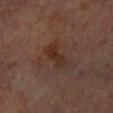This lesion was catalogued during total-body skin photography and was not selected for biopsy. Approximately 4 mm at its widest. A 15 mm close-up extracted from a 3D total-body photography capture. A male subject approximately 65 years of age. The tile uses cross-polarized illumination. The lesion is located on the left forearm. Automated tile analysis of the lesion measured a border-irregularity rating of about 3.5/10, a color-variation rating of about 5/10, and peripheral color asymmetry of about 2.